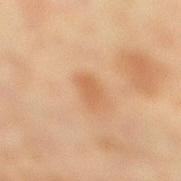No biopsy was performed on this lesion — it was imaged during a full skin examination and was not determined to be concerning.
A 15 mm close-up tile from a total-body photography series done for melanoma screening.
From the right lower leg.
A female patient, roughly 55 years of age.